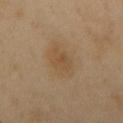| key | value |
|---|---|
| subject | male, aged 48 to 52 |
| illumination | cross-polarized illumination |
| size | about 4.5 mm |
| body site | the mid back |
| imaging modality | ~15 mm crop, total-body skin-cancer survey |